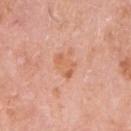Q: Is there a histopathology result?
A: imaged on a skin check; not biopsied
Q: What lighting was used for the tile?
A: white-light
Q: What is the imaging modality?
A: total-body-photography crop, ~15 mm field of view
Q: Lesion location?
A: the left upper arm
Q: Who is the patient?
A: male, aged 58 to 62
Q: Lesion size?
A: about 2.5 mm